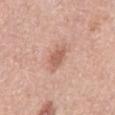Imaged during a routine full-body skin examination; the lesion was not biopsied and no histopathology is available.
The lesion is located on the left lower leg.
The total-body-photography lesion software estimated a classifier nevus-likeness of about 35/100 and a lesion-detection confidence of about 100/100.
A roughly 15 mm field-of-view crop from a total-body skin photograph.
A female patient, aged 63 to 67.
Longest diameter approximately 3 mm.
Captured under white-light illumination.